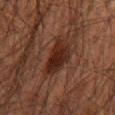This lesion was catalogued during total-body skin photography and was not selected for biopsy. Located on the mid back. Automated image analysis of the tile measured an outline eccentricity of about 0.85 (0 = round, 1 = elongated) and a symmetry-axis asymmetry near 0.3. The tile uses cross-polarized illumination. A roughly 15 mm field-of-view crop from a total-body skin photograph. The lesion's longest dimension is about 5 mm. A male patient, aged 58–62.